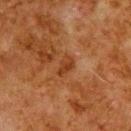Assessment: Part of a total-body skin-imaging series; this lesion was reviewed on a skin check and was not flagged for biopsy. Background: Automated tile analysis of the lesion measured a lesion color around L≈31 a*≈22 b*≈31 in CIELAB, a lesion–skin lightness drop of about 7, and a normalized border contrast of about 7. It also reported a border-irregularity index near 3/10, a within-lesion color-variation index near 1.5/10, and a peripheral color-asymmetry measure near 0.5. A 15 mm close-up extracted from a 3D total-body photography capture. From the upper back. The patient is a male aged 78–82. Captured under cross-polarized illumination. Measured at roughly 2.5 mm in maximum diameter.